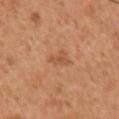| feature | finding |
|---|---|
| notes | imaged on a skin check; not biopsied |
| imaging modality | 15 mm crop, total-body photography |
| subject | male, aged approximately 55 |
| location | the front of the torso |